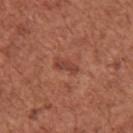No biopsy was performed on this lesion — it was imaged during a full skin examination and was not determined to be concerning. The tile uses white-light illumination. From the right upper arm. The recorded lesion diameter is about 3 mm. The subject is a female aged 48–52. A region of skin cropped from a whole-body photographic capture, roughly 15 mm wide. Automated tile analysis of the lesion measured a footprint of about 3.5 mm², a shape eccentricity near 0.9, and a symmetry-axis asymmetry near 0.3. It also reported border irregularity of about 3.5 on a 0–10 scale and peripheral color asymmetry of about 0.